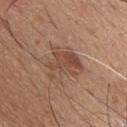<lesion>
<biopsy_status>not biopsied; imaged during a skin examination</biopsy_status>
<site>chest</site>
<patient>
  <sex>male</sex>
  <age_approx>60</age_approx>
</patient>
<image>
  <source>total-body photography crop</source>
  <field_of_view_mm>15</field_of_view_mm>
</image>
</lesion>